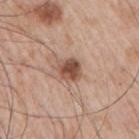* follow-up: catalogued during a skin exam; not biopsied
* patient: male, in their mid-60s
* TBP lesion metrics: a lesion color around L≈51 a*≈21 b*≈28 in CIELAB and roughly 14 lightness units darker than nearby skin; a border-irregularity rating of about 2/10 and radial color variation of about 1.5
* acquisition: ~15 mm crop, total-body skin-cancer survey
* site: the right upper arm
* diameter: ≈2.5 mm
* tile lighting: white-light